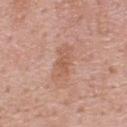No biopsy was performed on this lesion — it was imaged during a full skin examination and was not determined to be concerning. A male patient, aged around 55. A 15 mm crop from a total-body photograph taken for skin-cancer surveillance. The lesion is on the upper back. Automated image analysis of the tile measured an average lesion color of about L≈57 a*≈23 b*≈30 (CIELAB), a lesion–skin lightness drop of about 8, and a lesion-to-skin contrast of about 5.5 (normalized; higher = more distinct). The analysis additionally found a nevus-likeness score of about 0/100. Measured at roughly 3 mm in maximum diameter. Captured under white-light illumination.